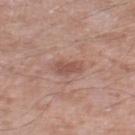The lesion was photographed on a routine skin check and not biopsied; there is no pathology result. About 3 mm across. Imaged with white-light lighting. Cropped from a whole-body photographic skin survey; the tile spans about 15 mm. From the left thigh. A male patient in their 60s.